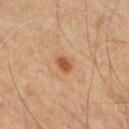Captured during whole-body skin photography for melanoma surveillance; the lesion was not biopsied. This is a cross-polarized tile. On the chest. The total-body-photography lesion software estimated a lesion area of about 3 mm², an eccentricity of roughly 0.7, and a symmetry-axis asymmetry near 0.25. The analysis additionally found an average lesion color of about L≈51 a*≈23 b*≈38 (CIELAB), about 11 CIELAB-L* units darker than the surrounding skin, and a normalized lesion–skin contrast near 9. It also reported a border-irregularity rating of about 2/10, a within-lesion color-variation index near 1.5/10, and peripheral color asymmetry of about 0.5. The analysis additionally found a detector confidence of about 100 out of 100 that the crop contains a lesion. Measured at roughly 2 mm in maximum diameter. A male patient, in their mid-50s. A roughly 15 mm field-of-view crop from a total-body skin photograph.